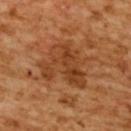Assessment: No biopsy was performed on this lesion — it was imaged during a full skin examination and was not determined to be concerning. Clinical summary: The recorded lesion diameter is about 6 mm. The tile uses cross-polarized illumination. From the upper back. A lesion tile, about 15 mm wide, cut from a 3D total-body photograph. The total-body-photography lesion software estimated a border-irregularity index near 5.5/10, internal color variation of about 5 on a 0–10 scale, and a peripheral color-asymmetry measure near 2. The analysis additionally found a classifier nevus-likeness of about 0/100 and a lesion-detection confidence of about 100/100. A female subject roughly 55 years of age.